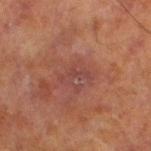Assessment:
No biopsy was performed on this lesion — it was imaged during a full skin examination and was not determined to be concerning.
Image and clinical context:
The subject is a male approximately 70 years of age. The tile uses cross-polarized illumination. The total-body-photography lesion software estimated a lesion area of about 4 mm² and two-axis asymmetry of about 0.6. It also reported border irregularity of about 7.5 on a 0–10 scale, internal color variation of about 0 on a 0–10 scale, and peripheral color asymmetry of about 0. The analysis additionally found an automated nevus-likeness rating near 0 out of 100. A 15 mm close-up tile from a total-body photography series done for melanoma screening. From the left lower leg.